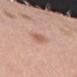Assessment: Part of a total-body skin-imaging series; this lesion was reviewed on a skin check and was not flagged for biopsy. Background: A 15 mm close-up extracted from a 3D total-body photography capture. Captured under white-light illumination. A female subject roughly 50 years of age. The lesion is on the right forearm. Approximately 2.5 mm at its widest.